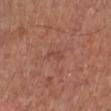Notes:
• notes — catalogued during a skin exam; not biopsied
• lesion size — about 2.5 mm
• illumination — white-light
• body site — the leg
• image — ~15 mm crop, total-body skin-cancer survey
• TBP lesion metrics — a shape eccentricity near 0.9 and two-axis asymmetry of about 0.4; a classifier nevus-likeness of about 0/100 and a detector confidence of about 100 out of 100 that the crop contains a lesion
• patient — male, roughly 70 years of age